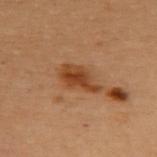biopsy status: imaged on a skin check; not biopsied
location: the upper back
patient: female, roughly 50 years of age
TBP lesion metrics: a footprint of about 8.5 mm², a shape eccentricity near 0.85, and two-axis asymmetry of about 0.25; a classifier nevus-likeness of about 90/100 and lesion-presence confidence of about 100/100
illumination: cross-polarized illumination
image: ~15 mm tile from a whole-body skin photo
diameter: ~4.5 mm (longest diameter)